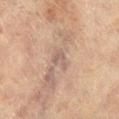Part of a total-body skin-imaging series; this lesion was reviewed on a skin check and was not flagged for biopsy. Imaged with cross-polarized lighting. An algorithmic analysis of the crop reported an area of roughly 3 mm², a shape eccentricity near 0.8, and two-axis asymmetry of about 0.5. The analysis additionally found a lesion color around L≈50 a*≈15 b*≈21 in CIELAB, a lesion–skin lightness drop of about 7, and a normalized lesion–skin contrast near 6. The software also gave a border-irregularity rating of about 5.5/10 and radial color variation of about 0.5. The analysis additionally found a lesion-detection confidence of about 60/100. This image is a 15 mm lesion crop taken from a total-body photograph. Located on the right lower leg. A female subject, aged around 80.